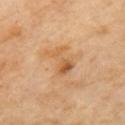Clinical impression:
Captured during whole-body skin photography for melanoma surveillance; the lesion was not biopsied.
Clinical summary:
On the mid back. Approximately 3.5 mm at its widest. A region of skin cropped from a whole-body photographic capture, roughly 15 mm wide. Automated image analysis of the tile measured a lesion area of about 6.5 mm² and a shape eccentricity near 0.8.